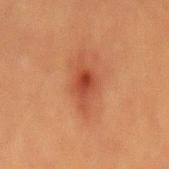No biopsy was performed on this lesion — it was imaged during a full skin examination and was not determined to be concerning.
A 15 mm crop from a total-body photograph taken for skin-cancer surveillance.
On the back.
A male subject about 40 years old.